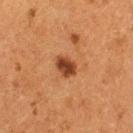Assessment:
Imaged during a routine full-body skin examination; the lesion was not biopsied and no histopathology is available.
Acquisition and patient details:
A female patient, in their 50s. The lesion is located on the left thigh. Imaged with cross-polarized lighting. Cropped from a total-body skin-imaging series; the visible field is about 15 mm. Automated image analysis of the tile measured a shape eccentricity near 0.65 and a shape-asymmetry score of about 0.15 (0 = symmetric). The software also gave border irregularity of about 1.5 on a 0–10 scale and a color-variation rating of about 2.5/10. And it measured a nevus-likeness score of about 95/100 and lesion-presence confidence of about 100/100. About 3 mm across.